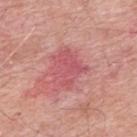Assessment: Captured during whole-body skin photography for melanoma surveillance; the lesion was not biopsied. Clinical summary: The subject is a male aged around 60. The tile uses white-light illumination. Located on the upper back. The lesion's longest dimension is about 4 mm. A close-up tile cropped from a whole-body skin photograph, about 15 mm across.